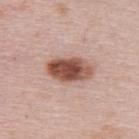notes = imaged on a skin check; not biopsied
anatomic site = the upper back
subject = female, in their mid- to late 60s
image = ~15 mm tile from a whole-body skin photo
size = ~5.5 mm (longest diameter)
automated lesion analysis = border irregularity of about 2 on a 0–10 scale, a within-lesion color-variation index near 8/10, and radial color variation of about 2.5; an automated nevus-likeness rating near 100 out of 100 and a detector confidence of about 100 out of 100 that the crop contains a lesion
lighting = white-light